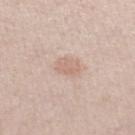| field | value |
|---|---|
| site | the left lower leg |
| size | ≈2.5 mm |
| subject | male, aged 58 to 62 |
| TBP lesion metrics | a lesion color around L≈66 a*≈18 b*≈26 in CIELAB, about 8 CIELAB-L* units darker than the surrounding skin, and a lesion-to-skin contrast of about 5 (normalized; higher = more distinct); a classifier nevus-likeness of about 15/100 |
| image source | total-body-photography crop, ~15 mm field of view |
| lighting | white-light |